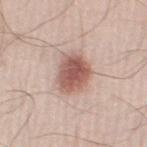Notes:
– notes — total-body-photography surveillance lesion; no biopsy
– subject — male, aged approximately 25
– location — the leg
– automated metrics — an area of roughly 12 mm², an eccentricity of roughly 0.65, and a shape-asymmetry score of about 0.2 (0 = symmetric); a mean CIELAB color near L≈57 a*≈21 b*≈25 and roughly 15 lightness units darker than nearby skin; an automated nevus-likeness rating near 100 out of 100 and a detector confidence of about 100 out of 100 that the crop contains a lesion
– imaging modality — total-body-photography crop, ~15 mm field of view
– lesion diameter — ~4.5 mm (longest diameter)
– tile lighting — white-light